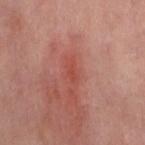Q: Is there a histopathology result?
A: imaged on a skin check; not biopsied
Q: Who is the patient?
A: female, approximately 50 years of age
Q: What is the anatomic site?
A: the left thigh
Q: What is the imaging modality?
A: total-body-photography crop, ~15 mm field of view